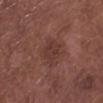Findings:
- follow-up: total-body-photography surveillance lesion; no biopsy
- subject: male, aged 53 to 57
- imaging modality: total-body-photography crop, ~15 mm field of view
- location: the left lower leg